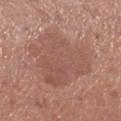Approximately 8 mm at its widest.
A female subject, aged 48 to 52.
Captured under white-light illumination.
From the leg.
A roughly 15 mm field-of-view crop from a total-body skin photograph.